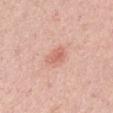Part of a total-body skin-imaging series; this lesion was reviewed on a skin check and was not flagged for biopsy.
Measured at roughly 2.5 mm in maximum diameter.
Located on the arm.
The lesion-visualizer software estimated a footprint of about 3.5 mm², an outline eccentricity of about 0.8 (0 = round, 1 = elongated), and a symmetry-axis asymmetry near 0.3. The analysis additionally found a border-irregularity index near 2.5/10, a color-variation rating of about 2/10, and a peripheral color-asymmetry measure near 1.
A 15 mm close-up extracted from a 3D total-body photography capture.
Captured under white-light illumination.
A male subject in their mid-60s.